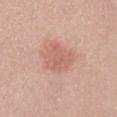{
  "biopsy_status": "not biopsied; imaged during a skin examination",
  "lighting": "white-light",
  "lesion_size": {
    "long_diameter_mm_approx": 4.0
  },
  "site": "front of the torso",
  "patient": {
    "sex": "female",
    "age_approx": 40
  },
  "image": {
    "source": "total-body photography crop",
    "field_of_view_mm": 15
  },
  "automated_metrics": {
    "area_mm2_approx": 11.0,
    "shape_asymmetry": 0.3,
    "border_irregularity_0_10": 3.0,
    "color_variation_0_10": 2.5,
    "peripheral_color_asymmetry": 1.0
  }
}